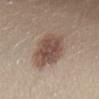* notes — catalogued during a skin exam; not biopsied
* size — about 5 mm
* image — ~15 mm tile from a whole-body skin photo
* TBP lesion metrics — a classifier nevus-likeness of about 70/100 and a lesion-detection confidence of about 100/100
* patient — female, aged 38 to 42
* site — the lower back
* lighting — white-light illumination